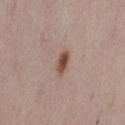Image and clinical context:
The lesion-visualizer software estimated border irregularity of about 2 on a 0–10 scale, a within-lesion color-variation index near 4.5/10, and a peripheral color-asymmetry measure near 1.5. The lesion is on the lower back. The subject is a female aged around 40. A roughly 15 mm field-of-view crop from a total-body skin photograph. The tile uses white-light illumination.